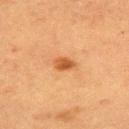The lesion is located on the upper back.
The patient is a female in their mid-50s.
A close-up tile cropped from a whole-body skin photograph, about 15 mm across.